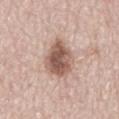Findings:
* follow-up: catalogued during a skin exam; not biopsied
* anatomic site: the back
* subject: male, approximately 65 years of age
* image: total-body-photography crop, ~15 mm field of view